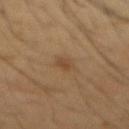The lesion was tiled from a total-body skin photograph and was not biopsied. A male patient, approximately 45 years of age. This image is a 15 mm lesion crop taken from a total-body photograph. The lesion is on the chest. The tile uses cross-polarized illumination.